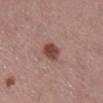Clinical impression:
Imaged during a routine full-body skin examination; the lesion was not biopsied and no histopathology is available.
Acquisition and patient details:
Captured under white-light illumination. The total-body-photography lesion software estimated a lesion color around L≈45 a*≈22 b*≈24 in CIELAB, about 13 CIELAB-L* units darker than the surrounding skin, and a lesion-to-skin contrast of about 10 (normalized; higher = more distinct). And it measured border irregularity of about 1.5 on a 0–10 scale, internal color variation of about 2.5 on a 0–10 scale, and peripheral color asymmetry of about 1. On the right lower leg. The patient is a female aged 48–52. A 15 mm close-up extracted from a 3D total-body photography capture. Longest diameter approximately 3 mm.